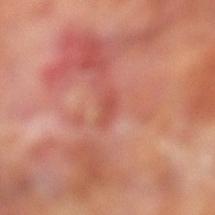A male subject, aged 68–72.
The lesion is located on the left lower leg.
The total-body-photography lesion software estimated a footprint of about 3 mm² and an eccentricity of roughly 0.85. The analysis additionally found an average lesion color of about L≈51 a*≈31 b*≈30 (CIELAB), about 7 CIELAB-L* units darker than the surrounding skin, and a normalized lesion–skin contrast near 5. It also reported a border-irregularity index near 3/10, a within-lesion color-variation index near 0.5/10, and radial color variation of about 0. It also reported a classifier nevus-likeness of about 0/100 and a lesion-detection confidence of about 100/100.
A lesion tile, about 15 mm wide, cut from a 3D total-body photograph.
This is a cross-polarized tile.